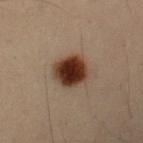Captured during whole-body skin photography for melanoma surveillance; the lesion was not biopsied.
The tile uses cross-polarized illumination.
A male patient in their mid-50s.
An algorithmic analysis of the crop reported a lesion area of about 12 mm², an eccentricity of roughly 0.45, and a symmetry-axis asymmetry near 0.15.
This image is a 15 mm lesion crop taken from a total-body photograph.
On the left forearm.